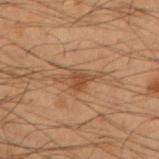Q: Was a biopsy performed?
A: imaged on a skin check; not biopsied
Q: Illumination type?
A: cross-polarized illumination
Q: How large is the lesion?
A: ~4.5 mm (longest diameter)
Q: What is the anatomic site?
A: the left forearm
Q: What are the patient's age and sex?
A: male, roughly 55 years of age
Q: What is the imaging modality?
A: ~15 mm crop, total-body skin-cancer survey